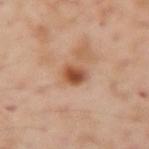No biopsy was performed on this lesion — it was imaged during a full skin examination and was not determined to be concerning. From the left upper arm. The patient is a male approximately 55 years of age. A region of skin cropped from a whole-body photographic capture, roughly 15 mm wide. This is a cross-polarized tile. About 2.5 mm across.